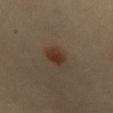Imaged during a routine full-body skin examination; the lesion was not biopsied and no histopathology is available.
The lesion's longest dimension is about 3.5 mm.
Automated image analysis of the tile measured a color-variation rating of about 4/10 and a peripheral color-asymmetry measure near 1.5. And it measured a classifier nevus-likeness of about 100/100.
Cropped from a whole-body photographic skin survey; the tile spans about 15 mm.
The lesion is on the chest.
A female patient aged approximately 65.
This is a cross-polarized tile.